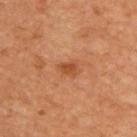Part of a total-body skin-imaging series; this lesion was reviewed on a skin check and was not flagged for biopsy. This is a cross-polarized tile. A male patient aged 58 to 62. The lesion is on the upper back. This image is a 15 mm lesion crop taken from a total-body photograph. About 2 mm across. Automated image analysis of the tile measured an area of roughly 2.5 mm² and a symmetry-axis asymmetry near 0.3. And it measured a mean CIELAB color near L≈47 a*≈27 b*≈38 and a lesion-to-skin contrast of about 7 (normalized; higher = more distinct). And it measured a border-irregularity rating of about 3/10 and peripheral color asymmetry of about 0.5. It also reported an automated nevus-likeness rating near 45 out of 100 and a detector confidence of about 100 out of 100 that the crop contains a lesion.